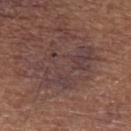<case>
<biopsy_status>not biopsied; imaged during a skin examination</biopsy_status>
<image>
  <source>total-body photography crop</source>
  <field_of_view_mm>15</field_of_view_mm>
</image>
<patient>
  <sex>male</sex>
  <age_approx>65</age_approx>
</patient>
<site>left upper arm</site>
<lesion_size>
  <long_diameter_mm_approx>7.0</long_diameter_mm_approx>
</lesion_size>
</case>